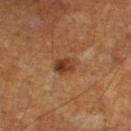<record>
  <biopsy_status>not biopsied; imaged during a skin examination</biopsy_status>
  <patient>
    <sex>male</sex>
    <age_approx>60</age_approx>
  </patient>
  <automated_metrics>
    <nevus_likeness_0_100>90</nevus_likeness_0_100>
    <lesion_detection_confidence_0_100>100</lesion_detection_confidence_0_100>
  </automated_metrics>
  <image>
    <source>total-body photography crop</source>
    <field_of_view_mm>15</field_of_view_mm>
  </image>
  <site>left lower leg</site>
</record>